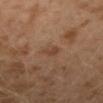A 15 mm close-up tile from a total-body photography series done for melanoma screening. The tile uses cross-polarized illumination. A male patient, about 30 years old. The recorded lesion diameter is about 2.5 mm. The lesion is located on the left lower leg.